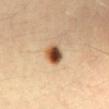Q: What did automated image analysis measure?
A: a footprint of about 5.5 mm², an outline eccentricity of about 0.6 (0 = round, 1 = elongated), and two-axis asymmetry of about 0.15; a lesion–skin lightness drop of about 21 and a normalized lesion–skin contrast near 13.5; a nevus-likeness score of about 100/100 and lesion-presence confidence of about 100/100
Q: What kind of image is this?
A: ~15 mm crop, total-body skin-cancer survey
Q: Where on the body is the lesion?
A: the abdomen
Q: What are the patient's age and sex?
A: male, aged around 40
Q: What is the lesion's diameter?
A: ≈3 mm
Q: What lighting was used for the tile?
A: cross-polarized illumination